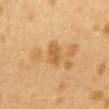This lesion was catalogued during total-body skin photography and was not selected for biopsy. Longest diameter approximately 3 mm. From the mid back. Captured under cross-polarized illumination. A female patient, aged approximately 40. A region of skin cropped from a whole-body photographic capture, roughly 15 mm wide. Automated tile analysis of the lesion measured a footprint of about 4 mm² and a shape-asymmetry score of about 0.2 (0 = symmetric). The software also gave a mean CIELAB color near L≈47 a*≈17 b*≈36 and about 8 CIELAB-L* units darker than the surrounding skin.